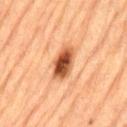<case>
  <image>
    <source>total-body photography crop</source>
    <field_of_view_mm>15</field_of_view_mm>
  </image>
  <site>lower back</site>
  <patient>
    <sex>male</sex>
    <age_approx>85+</age_approx>
  </patient>
  <lesion_size>
    <long_diameter_mm_approx>4.0</long_diameter_mm_approx>
  </lesion_size>
  <lighting>cross-polarized</lighting>
</case>